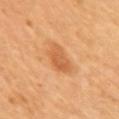Context: This image is a 15 mm lesion crop taken from a total-body photograph. The patient is a female roughly 55 years of age. Located on the right upper arm. An algorithmic analysis of the crop reported a lesion area of about 8.5 mm² and a shape eccentricity near 0.8.